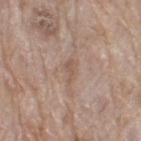<case>
<biopsy_status>not biopsied; imaged during a skin examination</biopsy_status>
<lighting>white-light</lighting>
<patient>
  <sex>female</sex>
  <age_approx>75</age_approx>
</patient>
<image>
  <source>total-body photography crop</source>
  <field_of_view_mm>15</field_of_view_mm>
</image>
<site>right thigh</site>
<lesion_size>
  <long_diameter_mm_approx>3.0</long_diameter_mm_approx>
</lesion_size>
</case>